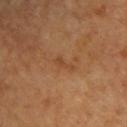Impression:
The lesion was tiled from a total-body skin photograph and was not biopsied.
Acquisition and patient details:
The patient is aged 58–62. On the upper back. A close-up tile cropped from a whole-body skin photograph, about 15 mm across. Approximately 2.5 mm at its widest.